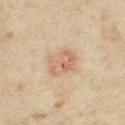Assessment: The lesion was tiled from a total-body skin photograph and was not biopsied. Clinical summary: On the right thigh. A roughly 15 mm field-of-view crop from a total-body skin photograph. About 4 mm across. Captured under cross-polarized illumination. The subject is a female about 35 years old.